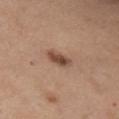The lesion was photographed on a routine skin check and not biopsied; there is no pathology result. The patient is a female in their 40s. About 3.5 mm across. On the right upper arm. A 15 mm crop from a total-body photograph taken for skin-cancer surveillance. The tile uses white-light illumination.